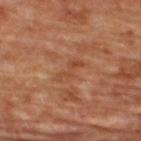Q: Was this lesion biopsied?
A: imaged on a skin check; not biopsied
Q: How was this image acquired?
A: total-body-photography crop, ~15 mm field of view
Q: Who is the patient?
A: female, roughly 45 years of age
Q: Lesion size?
A: ≈3.5 mm
Q: How was the tile lit?
A: cross-polarized illumination
Q: Automated lesion metrics?
A: a lesion–skin lightness drop of about 6 and a normalized border contrast of about 5.5; a classifier nevus-likeness of about 0/100 and a lesion-detection confidence of about 100/100
Q: Where on the body is the lesion?
A: the upper back